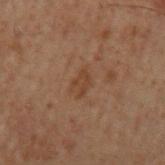No biopsy was performed on this lesion — it was imaged during a full skin examination and was not determined to be concerning. The lesion is on the left upper arm. A male subject roughly 60 years of age. The total-body-photography lesion software estimated a footprint of about 2.5 mm², an outline eccentricity of about 0.9 (0 = round, 1 = elongated), and a symmetry-axis asymmetry near 0.35. It also reported an average lesion color of about L≈30 a*≈16 b*≈25 (CIELAB), about 5 CIELAB-L* units darker than the surrounding skin, and a normalized lesion–skin contrast near 6. It also reported a classifier nevus-likeness of about 0/100 and lesion-presence confidence of about 100/100. A roughly 15 mm field-of-view crop from a total-body skin photograph.